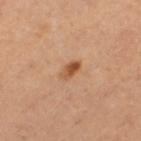Case summary:
* workup · total-body-photography surveillance lesion; no biopsy